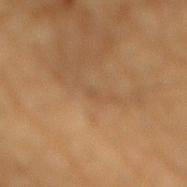The lesion was tiled from a total-body skin photograph and was not biopsied.
Captured under cross-polarized illumination.
Automated tile analysis of the lesion measured a lesion area of about 1 mm² and a shape-asymmetry score of about 0.4 (0 = symmetric). And it measured border irregularity of about 3 on a 0–10 scale, a color-variation rating of about 0/10, and a peripheral color-asymmetry measure near 0.
The subject is a male roughly 85 years of age or older.
A close-up tile cropped from a whole-body skin photograph, about 15 mm across.
On the mid back.
Longest diameter approximately 1 mm.